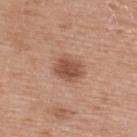Case summary:
– biopsy status — no biopsy performed (imaged during a skin exam)
– tile lighting — white-light illumination
– lesion diameter — ≈3.5 mm
– subject — female, aged 58 to 62
– image — ~15 mm tile from a whole-body skin photo
– location — the upper back
– TBP lesion metrics — an area of roughly 9 mm² and an outline eccentricity of about 0.6 (0 = round, 1 = elongated); a nevus-likeness score of about 85/100 and lesion-presence confidence of about 100/100